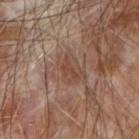Assessment: This lesion was catalogued during total-body skin photography and was not selected for biopsy. Image and clinical context: A 15 mm close-up tile from a total-body photography series done for melanoma screening. The patient is a male approximately 70 years of age. The tile uses cross-polarized illumination. On the arm. Approximately 3 mm at its widest.